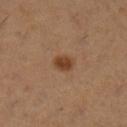Impression:
The lesion was tiled from a total-body skin photograph and was not biopsied.
Clinical summary:
A female patient, aged 53–57. The lesion is located on the left lower leg. Approximately 2.5 mm at its widest. The tile uses cross-polarized illumination. Cropped from a whole-body photographic skin survey; the tile spans about 15 mm.